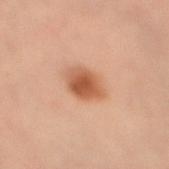Q: Is there a histopathology result?
A: no biopsy performed (imaged during a skin exam)
Q: Lesion location?
A: the right thigh
Q: How was this image acquired?
A: ~15 mm tile from a whole-body skin photo
Q: Who is the patient?
A: female, in their mid-30s
Q: How was the tile lit?
A: cross-polarized illumination